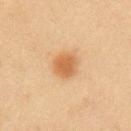An algorithmic analysis of the crop reported a border-irregularity index near 1.5/10 and radial color variation of about 0.5. Captured under cross-polarized illumination. A roughly 15 mm field-of-view crop from a total-body skin photograph. The subject is a male aged approximately 45. The lesion's longest dimension is about 3 mm. On the left upper arm.